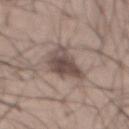biopsy status = catalogued during a skin exam; not biopsied | image source = total-body-photography crop, ~15 mm field of view | location = the abdomen | illumination = white-light illumination | patient = male, about 25 years old | lesion diameter = about 4.5 mm.